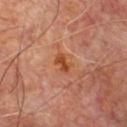| feature | finding |
|---|---|
| workup | catalogued during a skin exam; not biopsied |